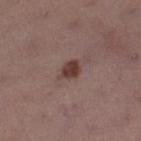No biopsy was performed on this lesion — it was imaged during a full skin examination and was not determined to be concerning. The patient is a female aged 53 to 57. Captured under white-light illumination. From the right lower leg. A close-up tile cropped from a whole-body skin photograph, about 15 mm across. Longest diameter approximately 3 mm.